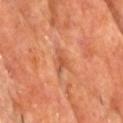{
  "biopsy_status": "not biopsied; imaged during a skin examination",
  "site": "upper back",
  "patient": {
    "sex": "male",
    "age_approx": 60
  },
  "automated_metrics": {
    "border_irregularity_0_10": 4.0,
    "color_variation_0_10": 2.5,
    "peripheral_color_asymmetry": 0.5,
    "nevus_likeness_0_100": 0,
    "lesion_detection_confidence_0_100": 95
  },
  "lighting": "cross-polarized",
  "image": {
    "source": "total-body photography crop",
    "field_of_view_mm": 15
  },
  "lesion_size": {
    "long_diameter_mm_approx": 2.5
  }
}